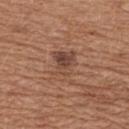Findings:
• workup — imaged on a skin check; not biopsied
• image — ~15 mm tile from a whole-body skin photo
• location — the upper back
• subject — female, aged approximately 55